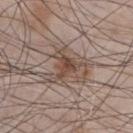The lesion was tiled from a total-body skin photograph and was not biopsied.
A male subject in their mid- to late 50s.
The recorded lesion diameter is about 2.5 mm.
Automated image analysis of the tile measured a shape eccentricity near 0.7 and a shape-asymmetry score of about 0.55 (0 = symmetric).
This is a white-light tile.
Located on the chest.
A close-up tile cropped from a whole-body skin photograph, about 15 mm across.